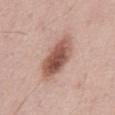Measured at roughly 6 mm in maximum diameter. A region of skin cropped from a whole-body photographic capture, roughly 15 mm wide. From the mid back. A male patient, about 55 years old. Imaged with white-light lighting.